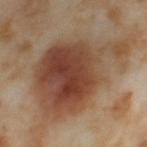Findings:
* automated lesion analysis · a shape eccentricity near 0.85 and two-axis asymmetry of about 0.4
* location · the left thigh
* lesion diameter · ~13.5 mm (longest diameter)
* patient · female, in their mid- to late 50s
* imaging modality · total-body-photography crop, ~15 mm field of view
* illumination · cross-polarized